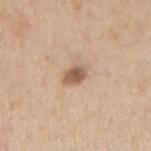Imaged during a routine full-body skin examination; the lesion was not biopsied and no histopathology is available.
The subject is a male about 55 years old.
Located on the right upper arm.
Imaged with white-light lighting.
Automated image analysis of the tile measured a footprint of about 4 mm² and a shape eccentricity near 0.7. And it measured border irregularity of about 2.5 on a 0–10 scale. The software also gave a classifier nevus-likeness of about 90/100 and a lesion-detection confidence of about 100/100.
A roughly 15 mm field-of-view crop from a total-body skin photograph.